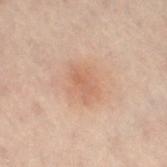Assessment:
Recorded during total-body skin imaging; not selected for excision or biopsy.
Clinical summary:
The patient is a male aged around 50. The lesion is located on the left leg. A 15 mm crop from a total-body photograph taken for skin-cancer surveillance.